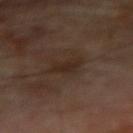{
  "lighting": "cross-polarized",
  "lesion_size": {
    "long_diameter_mm_approx": 3.5
  },
  "patient": {
    "sex": "male",
    "age_approx": 70
  },
  "site": "right forearm",
  "image": {
    "source": "total-body photography crop",
    "field_of_view_mm": 15
  }
}